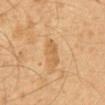This lesion was catalogued during total-body skin photography and was not selected for biopsy. The lesion is located on the abdomen. The tile uses cross-polarized illumination. Longest diameter approximately 3.5 mm. The patient is a male aged 48 to 52. Cropped from a total-body skin-imaging series; the visible field is about 15 mm.